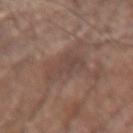The lesion was photographed on a routine skin check and not biopsied; there is no pathology result. Located on the right forearm. A male subject approximately 60 years of age. Cropped from a total-body skin-imaging series; the visible field is about 15 mm.